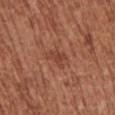follow-up=no biopsy performed (imaged during a skin exam) | image=~15 mm tile from a whole-body skin photo | patient=female, in their mid-70s | site=the left upper arm.